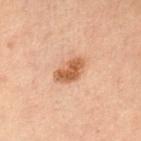Clinical impression: No biopsy was performed on this lesion — it was imaged during a full skin examination and was not determined to be concerning. Context: On the front of the torso. About 4 mm across. The tile uses cross-polarized illumination. A male subject aged around 60. This image is a 15 mm lesion crop taken from a total-body photograph.